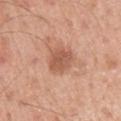Q: Was this lesion biopsied?
A: catalogued during a skin exam; not biopsied
Q: Patient demographics?
A: male, in their mid-50s
Q: Illumination type?
A: white-light
Q: What is the imaging modality?
A: ~15 mm tile from a whole-body skin photo
Q: What is the anatomic site?
A: the right upper arm
Q: What is the lesion's diameter?
A: about 3.5 mm